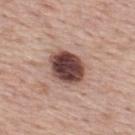Captured during whole-body skin photography for melanoma surveillance; the lesion was not biopsied. An algorithmic analysis of the crop reported a lesion area of about 13 mm², a shape eccentricity near 0.7, and a shape-asymmetry score of about 0.15 (0 = symmetric). The analysis additionally found a border-irregularity index near 1.5/10 and a within-lesion color-variation index near 5.5/10. It also reported a lesion-detection confidence of about 100/100. The lesion is located on the back. Imaged with white-light lighting. The patient is a male in their mid-70s. A 15 mm close-up extracted from a 3D total-body photography capture. About 4.5 mm across.